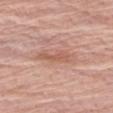The lesion was tiled from a total-body skin photograph and was not biopsied. A 15 mm crop from a total-body photograph taken for skin-cancer surveillance. From the left forearm. A female patient, aged approximately 65. An algorithmic analysis of the crop reported a footprint of about 6.5 mm² and a shape-asymmetry score of about 0.3 (0 = symmetric). It also reported a nevus-likeness score of about 5/100 and a lesion-detection confidence of about 100/100. The tile uses white-light illumination. Measured at roughly 4.5 mm in maximum diameter.